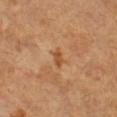<record>
  <biopsy_status>not biopsied; imaged during a skin examination</biopsy_status>
  <site>right lower leg</site>
  <image>
    <source>total-body photography crop</source>
    <field_of_view_mm>15</field_of_view_mm>
  </image>
  <lighting>cross-polarized</lighting>
  <patient>
    <sex>female</sex>
    <age_approx>55</age_approx>
  </patient>
  <lesion_size>
    <long_diameter_mm_approx>2.5</long_diameter_mm_approx>
  </lesion_size>
</record>